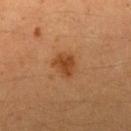The lesion was tiled from a total-body skin photograph and was not biopsied.
Imaged with cross-polarized lighting.
Located on the right upper arm.
Automated image analysis of the tile measured an automated nevus-likeness rating near 95 out of 100 and a detector confidence of about 100 out of 100 that the crop contains a lesion.
The patient is a female in their 40s.
Cropped from a total-body skin-imaging series; the visible field is about 15 mm.